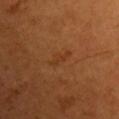<tbp_lesion>
  <biopsy_status>not biopsied; imaged during a skin examination</biopsy_status>
  <image>
    <source>total-body photography crop</source>
    <field_of_view_mm>15</field_of_view_mm>
  </image>
  <lighting>cross-polarized</lighting>
  <patient>
    <sex>male</sex>
    <age_approx>60</age_approx>
  </patient>
  <automated_metrics>
    <eccentricity>0.95</eccentricity>
    <shape_asymmetry>0.4</shape_asymmetry>
    <border_irregularity_0_10>5.5</border_irregularity_0_10>
  </automated_metrics>
  <site>chest</site>
</tbp_lesion>